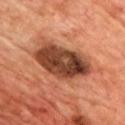Q: Is there a histopathology result?
A: catalogued during a skin exam; not biopsied
Q: What did automated image analysis measure?
A: a mean CIELAB color near L≈40 a*≈24 b*≈31, a lesion–skin lightness drop of about 18, and a normalized border contrast of about 13; a classifier nevus-likeness of about 0/100 and a lesion-detection confidence of about 100/100
Q: Who is the patient?
A: male, aged around 70
Q: What is the imaging modality?
A: 15 mm crop, total-body photography
Q: Lesion location?
A: the chest
Q: Illumination type?
A: cross-polarized illumination